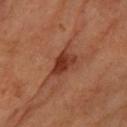{
  "biopsy_status": "not biopsied; imaged during a skin examination",
  "site": "left forearm",
  "lesion_size": {
    "long_diameter_mm_approx": 4.0
  },
  "patient": {
    "sex": "female",
    "age_approx": 65
  },
  "image": {
    "source": "total-body photography crop",
    "field_of_view_mm": 15
  },
  "lighting": "cross-polarized"
}